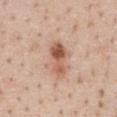Impression: This lesion was catalogued during total-body skin photography and was not selected for biopsy. Image and clinical context: The recorded lesion diameter is about 4.5 mm. A female subject, aged around 45. A 15 mm close-up extracted from a 3D total-body photography capture. The total-body-photography lesion software estimated a lesion color around L≈57 a*≈22 b*≈30 in CIELAB and a normalized lesion–skin contrast near 8.5. Imaged with white-light lighting. Located on the chest.